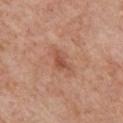Notes:
– biopsy status · catalogued during a skin exam; not biopsied
– diameter · ~3.5 mm (longest diameter)
– lighting · white-light illumination
– acquisition · total-body-photography crop, ~15 mm field of view
– subject · male, aged around 60
– location · the chest
– image-analysis metrics · a lesion-to-skin contrast of about 6 (normalized; higher = more distinct); a color-variation rating of about 2.5/10